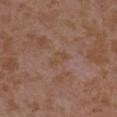workup = catalogued during a skin exam; not biopsied | location = the left upper arm | size = ≈2.5 mm | image-analysis metrics = an area of roughly 3 mm² and two-axis asymmetry of about 0.35; an average lesion color of about L≈47 a*≈18 b*≈29 (CIELAB), roughly 4 lightness units darker than nearby skin, and a normalized border contrast of about 5 | image source = total-body-photography crop, ~15 mm field of view | tile lighting = white-light illumination | subject = female, in their mid-30s.